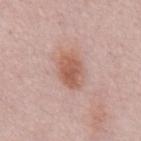Recorded during total-body skin imaging; not selected for excision or biopsy.
A region of skin cropped from a whole-body photographic capture, roughly 15 mm wide.
The total-body-photography lesion software estimated a lesion color around L≈58 a*≈23 b*≈28 in CIELAB, about 10 CIELAB-L* units darker than the surrounding skin, and a normalized lesion–skin contrast near 8. And it measured border irregularity of about 1.5 on a 0–10 scale. The analysis additionally found a classifier nevus-likeness of about 95/100 and a detector confidence of about 100 out of 100 that the crop contains a lesion.
Imaged with white-light lighting.
A male patient, aged 63–67.
The lesion is located on the front of the torso.
About 3.5 mm across.